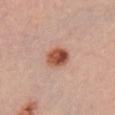{
  "biopsy_status": "not biopsied; imaged during a skin examination",
  "lesion_size": {
    "long_diameter_mm_approx": 3.0
  },
  "automated_metrics": {
    "border_irregularity_0_10": 1.5,
    "color_variation_0_10": 8.5,
    "peripheral_color_asymmetry": 3.5,
    "lesion_detection_confidence_0_100": 100
  },
  "site": "chest",
  "lighting": "white-light",
  "patient": {
    "sex": "female",
    "age_approx": 25
  },
  "image": {
    "source": "total-body photography crop",
    "field_of_view_mm": 15
  }
}